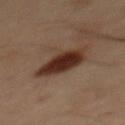The lesion was tiled from a total-body skin photograph and was not biopsied.